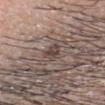This lesion was catalogued during total-body skin photography and was not selected for biopsy. About 2.5 mm across. From the head or neck. Imaged with white-light lighting. A male subject, aged approximately 35. A close-up tile cropped from a whole-body skin photograph, about 15 mm across.